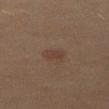{
  "biopsy_status": "not biopsied; imaged during a skin examination",
  "site": "left thigh",
  "image": {
    "source": "total-body photography crop",
    "field_of_view_mm": 15
  },
  "lighting": "cross-polarized",
  "lesion_size": {
    "long_diameter_mm_approx": 2.5
  },
  "patient": {
    "sex": "female",
    "age_approx": 60
  },
  "automated_metrics": {
    "border_irregularity_0_10": 2.0,
    "color_variation_0_10": 1.0,
    "peripheral_color_asymmetry": 0.5
  }
}